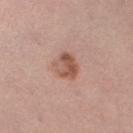workup — imaged on a skin check; not biopsied
subject — female, in their mid-30s
illumination — white-light
image — total-body-photography crop, ~15 mm field of view
automated lesion analysis — a footprint of about 7.5 mm² and an outline eccentricity of about 0.5 (0 = round, 1 = elongated); an average lesion color of about L≈55 a*≈22 b*≈28 (CIELAB) and roughly 11 lightness units darker than nearby skin; a border-irregularity index near 2/10, internal color variation of about 7 on a 0–10 scale, and peripheral color asymmetry of about 2.5
diameter — ~3.5 mm (longest diameter)
body site — the left lower leg